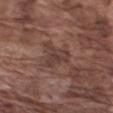Q: Is there a histopathology result?
A: catalogued during a skin exam; not biopsied
Q: What kind of image is this?
A: total-body-photography crop, ~15 mm field of view
Q: How was the tile lit?
A: white-light
Q: Lesion location?
A: the right upper arm
Q: What are the patient's age and sex?
A: male, aged 73–77